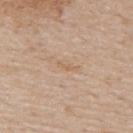workup: catalogued during a skin exam; not biopsied
diameter: about 2.5 mm
acquisition: total-body-photography crop, ~15 mm field of view
tile lighting: white-light
patient: male, in their mid-60s
site: the upper back